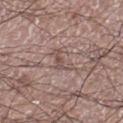Imaged during a routine full-body skin examination; the lesion was not biopsied and no histopathology is available.
The lesion is on the right lower leg.
The total-body-photography lesion software estimated a footprint of about 4 mm², an outline eccentricity of about 0.8 (0 = round, 1 = elongated), and a shape-asymmetry score of about 0.7 (0 = symmetric). It also reported a lesion color around L≈50 a*≈17 b*≈21 in CIELAB and about 8 CIELAB-L* units darker than the surrounding skin. It also reported border irregularity of about 8.5 on a 0–10 scale and internal color variation of about 2.5 on a 0–10 scale. The analysis additionally found an automated nevus-likeness rating near 0 out of 100 and lesion-presence confidence of about 85/100.
A lesion tile, about 15 mm wide, cut from a 3D total-body photograph.
A male patient, about 60 years old.